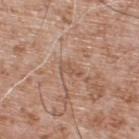Findings:
- biopsy status · no biopsy performed (imaged during a skin exam)
- acquisition · total-body-photography crop, ~15 mm field of view
- diameter · ~2.5 mm (longest diameter)
- anatomic site · the back
- TBP lesion metrics · a lesion color around L≈54 a*≈19 b*≈29 in CIELAB, roughly 7 lightness units darker than nearby skin, and a normalized border contrast of about 5.5; a border-irregularity rating of about 4/10 and internal color variation of about 0 on a 0–10 scale; a classifier nevus-likeness of about 0/100 and a lesion-detection confidence of about 55/100
- patient · male, in their mid- to late 50s